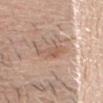workup = total-body-photography surveillance lesion; no biopsy
tile lighting = white-light illumination
size = about 4 mm
automated lesion analysis = an average lesion color of about L≈58 a*≈19 b*≈28 (CIELAB) and a lesion–skin lightness drop of about 8; a border-irregularity index near 5/10, a within-lesion color-variation index near 3.5/10, and radial color variation of about 1; a classifier nevus-likeness of about 0/100 and a lesion-detection confidence of about 100/100
location = the head or neck
patient = male, in their mid- to late 30s
image source = 15 mm crop, total-body photography